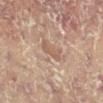| feature | finding |
|---|---|
| follow-up | total-body-photography surveillance lesion; no biopsy |
| patient | female, in their 80s |
| body site | the right leg |
| imaging modality | ~15 mm crop, total-body skin-cancer survey |